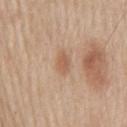| field | value |
|---|---|
| notes | total-body-photography surveillance lesion; no biopsy |
| acquisition | total-body-photography crop, ~15 mm field of view |
| location | the mid back |
| illumination | white-light |
| subject | male, roughly 60 years of age |